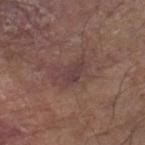follow-up: catalogued during a skin exam; not biopsied | patient: male, about 80 years old | site: the leg | image-analysis metrics: a shape-asymmetry score of about 0.45 (0 = symmetric); an average lesion color of about L≈39 a*≈18 b*≈17 (CIELAB) and a lesion-to-skin contrast of about 7 (normalized; higher = more distinct) | illumination: white-light | image: ~15 mm tile from a whole-body skin photo.